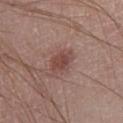Imaged during a routine full-body skin examination; the lesion was not biopsied and no histopathology is available. A 15 mm crop from a total-body photograph taken for skin-cancer surveillance. Located on the leg. A male patient, about 20 years old. Automated image analysis of the tile measured a mean CIELAB color near L≈45 a*≈21 b*≈22 and a lesion-to-skin contrast of about 7 (normalized; higher = more distinct). And it measured a border-irregularity index near 2.5/10, internal color variation of about 3 on a 0–10 scale, and radial color variation of about 1. The analysis additionally found a classifier nevus-likeness of about 65/100. Captured under white-light illumination.